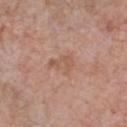{
  "biopsy_status": "not biopsied; imaged during a skin examination",
  "image": {
    "source": "total-body photography crop",
    "field_of_view_mm": 15
  },
  "lesion_size": {
    "long_diameter_mm_approx": 3.5
  },
  "patient": {
    "sex": "male",
    "age_approx": 60
  },
  "site": "chest",
  "automated_metrics": {
    "area_mm2_approx": 6.0,
    "eccentricity": 0.7,
    "shape_asymmetry": 0.4,
    "cielab_L": 56,
    "cielab_a": 20,
    "cielab_b": 29,
    "vs_skin_contrast_norm": 5.0,
    "border_irregularity_0_10": 4.0,
    "peripheral_color_asymmetry": 1.0
  }
}